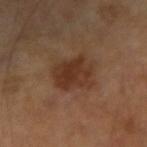Imaged during a routine full-body skin examination; the lesion was not biopsied and no histopathology is available. The subject is a male in their mid-60s. A lesion tile, about 15 mm wide, cut from a 3D total-body photograph. Located on the left arm.